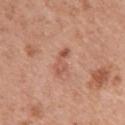The lesion is located on the left upper arm. Captured under white-light illumination. A female subject, approximately 40 years of age. Automated tile analysis of the lesion measured a border-irregularity index near 6/10, a within-lesion color-variation index near 0/10, and peripheral color asymmetry of about 0. The analysis additionally found a nevus-likeness score of about 0/100 and a lesion-detection confidence of about 100/100. A 15 mm close-up tile from a total-body photography series done for melanoma screening. The recorded lesion diameter is about 3.5 mm.